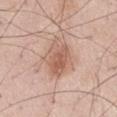The lesion was tiled from a total-body skin photograph and was not biopsied.
A region of skin cropped from a whole-body photographic capture, roughly 15 mm wide.
A male patient about 55 years old.
Located on the right thigh.
The total-body-photography lesion software estimated a mean CIELAB color near L≈60 a*≈20 b*≈29, roughly 10 lightness units darker than nearby skin, and a normalized border contrast of about 7. And it measured a border-irregularity rating of about 3/10 and radial color variation of about 2.
Approximately 5.5 mm at its widest.